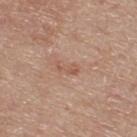The lesion was tiled from a total-body skin photograph and was not biopsied.
The lesion is located on the back.
Cropped from a total-body skin-imaging series; the visible field is about 15 mm.
Captured under white-light illumination.
Automated image analysis of the tile measured a border-irregularity index near 6.5/10. It also reported a classifier nevus-likeness of about 0/100 and a lesion-detection confidence of about 100/100.
A male subject, aged 73 to 77.